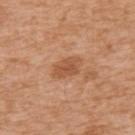No biopsy was performed on this lesion — it was imaged during a full skin examination and was not determined to be concerning. A male patient roughly 75 years of age. This image is a 15 mm lesion crop taken from a total-body photograph. The lesion is located on the upper back.